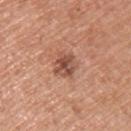workup: total-body-photography surveillance lesion; no biopsy
body site: the left upper arm
subject: male, aged 53–57
lighting: white-light
automated lesion analysis: a shape eccentricity near 0.6 and two-axis asymmetry of about 0.25; a lesion color around L≈50 a*≈24 b*≈29 in CIELAB and a normalized lesion–skin contrast near 8.5; a border-irregularity index near 2.5/10 and a peripheral color-asymmetry measure near 2
image: ~15 mm tile from a whole-body skin photo
lesion size: about 3 mm